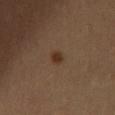{"biopsy_status": "not biopsied; imaged during a skin examination", "patient": {"sex": "female", "age_approx": 70}, "lesion_size": {"long_diameter_mm_approx": 2.0}, "image": {"source": "total-body photography crop", "field_of_view_mm": 15}, "site": "left thigh", "lighting": "cross-polarized"}